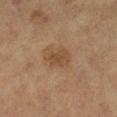The lesion was tiled from a total-body skin photograph and was not biopsied. Measured at roughly 3 mm in maximum diameter. A roughly 15 mm field-of-view crop from a total-body skin photograph. The lesion is on the right lower leg. A female patient aged around 60. This is a cross-polarized tile.